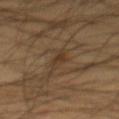Imaged during a routine full-body skin examination; the lesion was not biopsied and no histopathology is available.
Longest diameter approximately 4 mm.
A male subject, aged 58–62.
A roughly 15 mm field-of-view crop from a total-body skin photograph.
Imaged with cross-polarized lighting.
On the abdomen.